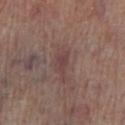This lesion was catalogued during total-body skin photography and was not selected for biopsy. A close-up tile cropped from a whole-body skin photograph, about 15 mm across. Approximately 3 mm at its widest. A male subject, aged around 70. Imaged with white-light lighting. Located on the right lower leg.